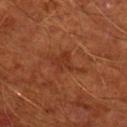biopsy_status: not biopsied; imaged during a skin examination
patient:
  sex: male
  age_approx: 65
image:
  source: total-body photography crop
  field_of_view_mm: 15
lighting: cross-polarized
site: right upper arm
automated_metrics:
  area_mm2_approx: 6.5
  shape_asymmetry: 0.3
  nevus_likeness_0_100: 0
  lesion_detection_confidence_0_100: 100
lesion_size:
  long_diameter_mm_approx: 4.5